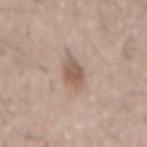• notes: catalogued during a skin exam; not biopsied
• image: ~15 mm tile from a whole-body skin photo
• patient: male, in their mid-60s
• location: the mid back